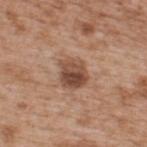Impression:
This lesion was catalogued during total-body skin photography and was not selected for biopsy.
Image and clinical context:
The recorded lesion diameter is about 3.5 mm. A male subject, aged approximately 65. A roughly 15 mm field-of-view crop from a total-body skin photograph. The tile uses white-light illumination. From the upper back. The lesion-visualizer software estimated a footprint of about 8.5 mm², an outline eccentricity of about 0.45 (0 = round, 1 = elongated), and a shape-asymmetry score of about 0.15 (0 = symmetric). And it measured a lesion-to-skin contrast of about 9 (normalized; higher = more distinct). The software also gave a nevus-likeness score of about 80/100 and a lesion-detection confidence of about 100/100.